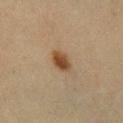The lesion was photographed on a routine skin check and not biopsied; there is no pathology result.
Imaged with cross-polarized lighting.
The total-body-photography lesion software estimated a shape eccentricity near 0.8 and a shape-asymmetry score of about 0.15 (0 = symmetric). The software also gave about 11 CIELAB-L* units darker than the surrounding skin and a normalized lesion–skin contrast near 10. The software also gave border irregularity of about 1.5 on a 0–10 scale, internal color variation of about 3.5 on a 0–10 scale, and peripheral color asymmetry of about 1.
From the right lower leg.
A female subject aged 53–57.
Cropped from a whole-body photographic skin survey; the tile spans about 15 mm.